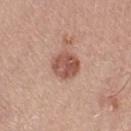site = the leg | imaging modality = total-body-photography crop, ~15 mm field of view | lesion diameter = ≈3 mm | patient = female, aged 53–57 | lighting = white-light illumination.